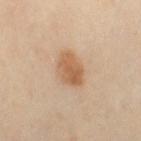The lesion was tiled from a total-body skin photograph and was not biopsied. The lesion is located on the right thigh. A female subject aged 48–52. Captured under cross-polarized illumination. A roughly 15 mm field-of-view crop from a total-body skin photograph.